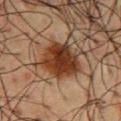Imaged during a routine full-body skin examination; the lesion was not biopsied and no histopathology is available. The lesion is on the chest. The subject is a male in their 60s. A 15 mm crop from a total-body photograph taken for skin-cancer surveillance.